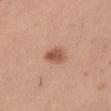Notes:
- workup: catalogued during a skin exam; not biopsied